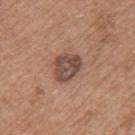Captured during whole-body skin photography for melanoma surveillance; the lesion was not biopsied. A region of skin cropped from a whole-body photographic capture, roughly 15 mm wide. Automated image analysis of the tile measured a border-irregularity index near 2/10, a color-variation rating of about 4.5/10, and peripheral color asymmetry of about 1.5. And it measured an automated nevus-likeness rating near 35 out of 100. A male patient, about 75 years old. About 3.5 mm across. This is a white-light tile. The lesion is on the left upper arm.